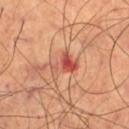This lesion was catalogued during total-body skin photography and was not selected for biopsy.
The patient is a male in their 70s.
A lesion tile, about 15 mm wide, cut from a 3D total-body photograph.
From the left thigh.
About 4 mm across.
This is a cross-polarized tile.